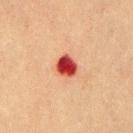{"biopsy_status": "not biopsied; imaged during a skin examination", "patient": {"sex": "male", "age_approx": 40}, "image": {"source": "total-body photography crop", "field_of_view_mm": 15}, "site": "front of the torso"}